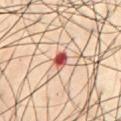Notes:
• biopsy status — no biopsy performed (imaged during a skin exam)
• illumination — cross-polarized illumination
• location — the chest
• imaging modality — 15 mm crop, total-body photography
• subject — male, approximately 50 years of age